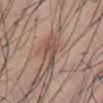Q: Is there a histopathology result?
A: total-body-photography surveillance lesion; no biopsy
Q: Who is the patient?
A: male, aged 48–52
Q: What kind of image is this?
A: ~15 mm crop, total-body skin-cancer survey
Q: Lesion location?
A: the abdomen